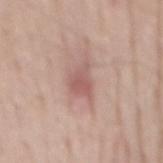workup: catalogued during a skin exam; not biopsied | patient: male, approximately 55 years of age | image source: 15 mm crop, total-body photography | site: the mid back.